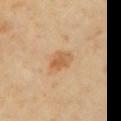The lesion was photographed on a routine skin check and not biopsied; there is no pathology result. Imaged with cross-polarized lighting. Cropped from a total-body skin-imaging series; the visible field is about 15 mm. The lesion is on the left upper arm. The subject is a female aged 58 to 62.